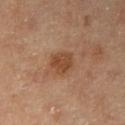Imaged during a routine full-body skin examination; the lesion was not biopsied and no histopathology is available. The patient is a female in their mid-60s. Captured under cross-polarized illumination. The lesion is located on the arm. A 15 mm crop from a total-body photograph taken for skin-cancer surveillance.